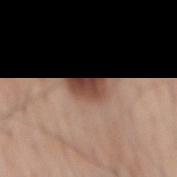Recorded during total-body skin imaging; not selected for excision or biopsy. A male subject aged 68–72. The lesion is located on the mid back. A 15 mm crop from a total-body photograph taken for skin-cancer surveillance.